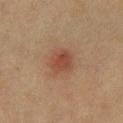| key | value |
|---|---|
| workup | total-body-photography surveillance lesion; no biopsy |
| image-analysis metrics | an area of roughly 7 mm² and a shape eccentricity near 0.5; a classifier nevus-likeness of about 95/100 and lesion-presence confidence of about 100/100 |
| lesion diameter | ~3.5 mm (longest diameter) |
| illumination | cross-polarized illumination |
| image | ~15 mm crop, total-body skin-cancer survey |
| patient | female, approximately 40 years of age |
| site | the chest |